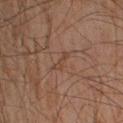Recorded during total-body skin imaging; not selected for excision or biopsy. On the right lower leg. A close-up tile cropped from a whole-body skin photograph, about 15 mm across. Automated tile analysis of the lesion measured a symmetry-axis asymmetry near 0.4. It also reported a lesion color around L≈34 a*≈15 b*≈23 in CIELAB and a normalized lesion–skin contrast near 5. A male subject, aged 43–47. This is a cross-polarized tile. Approximately 3 mm at its widest.